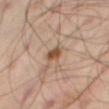Impression:
Captured during whole-body skin photography for melanoma surveillance; the lesion was not biopsied.
Context:
The patient is a male aged around 45. Automated image analysis of the tile measured a symmetry-axis asymmetry near 0.3. And it measured a border-irregularity index near 2/10, a color-variation rating of about 2/10, and radial color variation of about 1. It also reported an automated nevus-likeness rating near 80 out of 100 and a detector confidence of about 100 out of 100 that the crop contains a lesion. The lesion's longest dimension is about 2.5 mm. A close-up tile cropped from a whole-body skin photograph, about 15 mm across. Located on the right thigh.